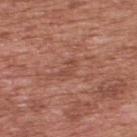Cropped from a total-body skin-imaging series; the visible field is about 15 mm.
A male patient, aged 68 to 72.
Located on the upper back.